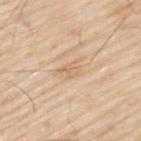Findings:
* workup — no biopsy performed (imaged during a skin exam)
* lighting — white-light
* acquisition — ~15 mm tile from a whole-body skin photo
* patient — male, approximately 80 years of age
* site — the back